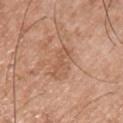No biopsy was performed on this lesion — it was imaged during a full skin examination and was not determined to be concerning. The subject is a male approximately 50 years of age. Imaged with white-light lighting. On the chest. A 15 mm close-up tile from a total-body photography series done for melanoma screening. The lesion's longest dimension is about 4.5 mm.